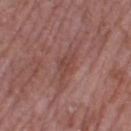{"biopsy_status": "not biopsied; imaged during a skin examination", "site": "left thigh", "lesion_size": {"long_diameter_mm_approx": 3.0}, "patient": {"sex": "female", "age_approx": 70}, "image": {"source": "total-body photography crop", "field_of_view_mm": 15}, "lighting": "white-light"}